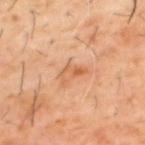Imaged during a routine full-body skin examination; the lesion was not biopsied and no histopathology is available.
The lesion-visualizer software estimated an area of roughly 3.5 mm², an outline eccentricity of about 0.9 (0 = round, 1 = elongated), and a symmetry-axis asymmetry near 0.6. And it measured an average lesion color of about L≈57 a*≈24 b*≈37 (CIELAB), a lesion–skin lightness drop of about 8, and a lesion-to-skin contrast of about 6 (normalized; higher = more distinct).
The patient is a male aged approximately 60.
The tile uses cross-polarized illumination.
A close-up tile cropped from a whole-body skin photograph, about 15 mm across.
Located on the upper back.
Approximately 3.5 mm at its widest.